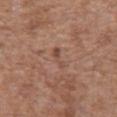Impression:
Captured during whole-body skin photography for melanoma surveillance; the lesion was not biopsied.
Image and clinical context:
Automated image analysis of the tile measured a nevus-likeness score of about 0/100 and a lesion-detection confidence of about 100/100. Measured at roughly 3 mm in maximum diameter. On the chest. A 15 mm close-up tile from a total-body photography series done for melanoma screening. The subject is a male aged approximately 65.